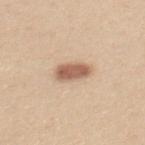Q: Where on the body is the lesion?
A: the upper back
Q: What is the imaging modality?
A: 15 mm crop, total-body photography
Q: What did automated image analysis measure?
A: an area of roughly 7 mm² and an eccentricity of roughly 0.8
Q: What lighting was used for the tile?
A: white-light
Q: What are the patient's age and sex?
A: female, in their mid-20s